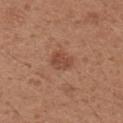notes=no biopsy performed (imaged during a skin exam).